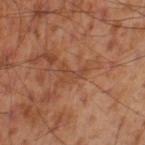Impression: The lesion was photographed on a routine skin check and not biopsied; there is no pathology result. Background: Measured at roughly 3 mm in maximum diameter. A 15 mm close-up tile from a total-body photography series done for melanoma screening. The patient is a male aged approximately 55. The lesion is located on the left thigh.